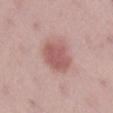Findings:
* follow-up: total-body-photography surveillance lesion; no biopsy